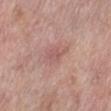Part of a total-body skin-imaging series; this lesion was reviewed on a skin check and was not flagged for biopsy.
The lesion is on the left lower leg.
This image is a 15 mm lesion crop taken from a total-body photograph.
A female patient, aged 58 to 62.